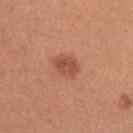Q: Is there a histopathology result?
A: no biopsy performed (imaged during a skin exam)
Q: What is the imaging modality?
A: total-body-photography crop, ~15 mm field of view
Q: Who is the patient?
A: female, about 25 years old
Q: Illumination type?
A: white-light
Q: What did automated image analysis measure?
A: border irregularity of about 1.5 on a 0–10 scale, internal color variation of about 2.5 on a 0–10 scale, and peripheral color asymmetry of about 1; a nevus-likeness score of about 90/100 and lesion-presence confidence of about 100/100
Q: What is the anatomic site?
A: the right upper arm